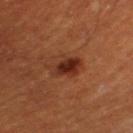Assessment: Part of a total-body skin-imaging series; this lesion was reviewed on a skin check and was not flagged for biopsy. Clinical summary: A male subject, aged 58–62. On the left thigh. A 15 mm close-up tile from a total-body photography series done for melanoma screening. About 4 mm across. This is a cross-polarized tile.